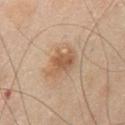This lesion was catalogued during total-body skin photography and was not selected for biopsy.
Located on the chest.
Cropped from a total-body skin-imaging series; the visible field is about 15 mm.
Imaged with white-light lighting.
A male subject aged approximately 75.